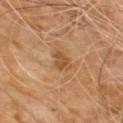<lesion>
  <biopsy_status>not biopsied; imaged during a skin examination</biopsy_status>
  <lighting>cross-polarized</lighting>
  <patient>
    <sex>male</sex>
    <age_approx>70</age_approx>
  </patient>
  <lesion_size>
    <long_diameter_mm_approx>3.0</long_diameter_mm_approx>
  </lesion_size>
  <site>chest</site>
  <automated_metrics>
    <area_mm2_approx>4.0</area_mm2_approx>
    <eccentricity>0.8</eccentricity>
    <shape_asymmetry>0.25</shape_asymmetry>
    <cielab_L>50</cielab_L>
    <cielab_a>21</cielab_a>
    <cielab_b>38</cielab_b>
    <vs_skin_contrast_norm>7.0</vs_skin_contrast_norm>
  </automated_metrics>
  <image>
    <source>total-body photography crop</source>
    <field_of_view_mm>15</field_of_view_mm>
  </image>
</lesion>